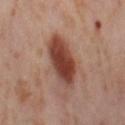notes: no biopsy performed (imaged during a skin exam) | diameter: ~6 mm (longest diameter) | image-analysis metrics: a lesion–skin lightness drop of about 15 and a lesion-to-skin contrast of about 11 (normalized; higher = more distinct) | image: 15 mm crop, total-body photography | subject: female, aged approximately 55 | illumination: cross-polarized illumination | anatomic site: the leg.